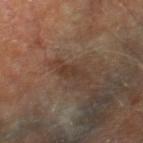{"patient": {"sex": "male", "age_approx": 70}, "site": "right thigh", "lighting": "cross-polarized", "image": {"source": "total-body photography crop", "field_of_view_mm": 15}}